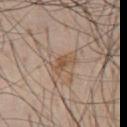Q: Was this lesion biopsied?
A: no biopsy performed (imaged during a skin exam)
Q: What is the lesion's diameter?
A: ≈3 mm
Q: Where on the body is the lesion?
A: the chest
Q: What kind of image is this?
A: ~15 mm crop, total-body skin-cancer survey
Q: Who is the patient?
A: male, roughly 60 years of age
Q: What lighting was used for the tile?
A: white-light